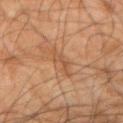Impression: This lesion was catalogued during total-body skin photography and was not selected for biopsy. Context: Captured under cross-polarized illumination. The lesion-visualizer software estimated a border-irregularity rating of about 7.5/10 and peripheral color asymmetry of about 0. The analysis additionally found an automated nevus-likeness rating near 0 out of 100 and a detector confidence of about 100 out of 100 that the crop contains a lesion. A male patient aged approximately 65. A 15 mm close-up tile from a total-body photography series done for melanoma screening. The recorded lesion diameter is about 3.5 mm. The lesion is on the arm.